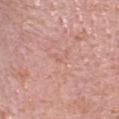Findings:
* notes: catalogued during a skin exam; not biopsied
* image source: 15 mm crop, total-body photography
* body site: the head or neck
* patient: female, about 50 years old
* lesion size: ≈1 mm